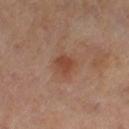The lesion was photographed on a routine skin check and not biopsied; there is no pathology result. This image is a 15 mm lesion crop taken from a total-body photograph. This is a cross-polarized tile. From the right thigh. The patient is a female about 50 years old.